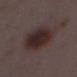{
  "biopsy_status": "not biopsied; imaged during a skin examination",
  "automated_metrics": {
    "area_mm2_approx": 22.0,
    "eccentricity": 0.7,
    "shape_asymmetry": 0.15,
    "cielab_L": 27,
    "cielab_a": 13,
    "cielab_b": 15,
    "vs_skin_darker_L": 9.0,
    "vs_skin_contrast_norm": 10.0,
    "nevus_likeness_0_100": 95,
    "lesion_detection_confidence_0_100": 100
  },
  "image": {
    "source": "total-body photography crop",
    "field_of_view_mm": 15
  },
  "site": "left lower leg",
  "patient": {
    "sex": "female",
    "age_approx": 30
  },
  "lesion_size": {
    "long_diameter_mm_approx": 6.0
  }
}